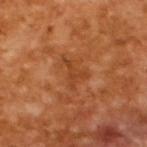The lesion was tiled from a total-body skin photograph and was not biopsied.
A close-up tile cropped from a whole-body skin photograph, about 15 mm across.
The tile uses cross-polarized illumination.
A male subject, roughly 65 years of age.
Measured at roughly 3.5 mm in maximum diameter.